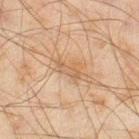The lesion was photographed on a routine skin check and not biopsied; there is no pathology result. A lesion tile, about 15 mm wide, cut from a 3D total-body photograph. A male patient, aged around 45. On the right thigh.